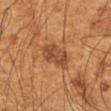<record>
<biopsy_status>not biopsied; imaged during a skin examination</biopsy_status>
<image>
  <source>total-body photography crop</source>
  <field_of_view_mm>15</field_of_view_mm>
</image>
<automated_metrics>
  <cielab_L>47</cielab_L>
  <cielab_a>24</cielab_a>
  <cielab_b>36</cielab_b>
  <vs_skin_contrast_norm>7.5</vs_skin_contrast_norm>
  <color_variation_0_10>3.5</color_variation_0_10>
  <peripheral_color_asymmetry>1.0</peripheral_color_asymmetry>
</automated_metrics>
<lighting>cross-polarized</lighting>
<lesion_size>
  <long_diameter_mm_approx>3.5</long_diameter_mm_approx>
</lesion_size>
<site>right upper arm</site>
<patient>
  <sex>male</sex>
  <age_approx>60</age_approx>
</patient>
</record>